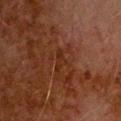notes — total-body-photography surveillance lesion; no biopsy | acquisition — 15 mm crop, total-body photography | patient — male, in their 80s | body site — the upper back.